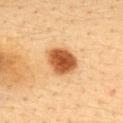Clinical impression:
The lesion was photographed on a routine skin check and not biopsied; there is no pathology result.
Image and clinical context:
Located on the upper back. The lesion's longest dimension is about 4 mm. A female patient, in their 40s. A roughly 15 mm field-of-view crop from a total-body skin photograph. The lesion-visualizer software estimated a lesion area of about 11 mm², an outline eccentricity of about 0.55 (0 = round, 1 = elongated), and a symmetry-axis asymmetry near 0.2. It also reported a within-lesion color-variation index near 4.5/10 and peripheral color asymmetry of about 1.5. The software also gave a nevus-likeness score of about 100/100 and lesion-presence confidence of about 100/100.